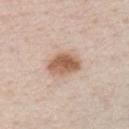{"site": "chest", "automated_metrics": {"cielab_L": 59, "cielab_a": 19, "cielab_b": 31, "vs_skin_contrast_norm": 9.5, "nevus_likeness_0_100": 95, "lesion_detection_confidence_0_100": 100}, "lesion_size": {"long_diameter_mm_approx": 4.0}, "image": {"source": "total-body photography crop", "field_of_view_mm": 15}, "lighting": "white-light", "patient": {"sex": "male", "age_approx": 70}}